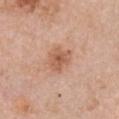<record>
  <biopsy_status>not biopsied; imaged during a skin examination</biopsy_status>
  <site>chest</site>
  <patient>
    <sex>female</sex>
    <age_approx>60</age_approx>
  </patient>
  <image>
    <source>total-body photography crop</source>
    <field_of_view_mm>15</field_of_view_mm>
  </image>
</record>